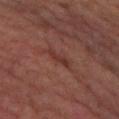No biopsy was performed on this lesion — it was imaged during a full skin examination and was not determined to be concerning.
A lesion tile, about 15 mm wide, cut from a 3D total-body photograph.
A female subject about 60 years old.
Imaged with cross-polarized lighting.
The recorded lesion diameter is about 2.5 mm.
From the left thigh.